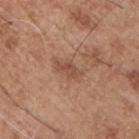| key | value |
|---|---|
| notes | imaged on a skin check; not biopsied |
| lesion size | about 2.5 mm |
| subject | male, in their mid-50s |
| anatomic site | the chest |
| tile lighting | white-light illumination |
| image source | 15 mm crop, total-body photography |